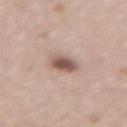Imaged during a routine full-body skin examination; the lesion was not biopsied and no histopathology is available.
Cropped from a whole-body photographic skin survey; the tile spans about 15 mm.
Approximately 3 mm at its widest.
From the back.
A female patient approximately 75 years of age.
Automated tile analysis of the lesion measured an average lesion color of about L≈54 a*≈17 b*≈24 (CIELAB), about 14 CIELAB-L* units darker than the surrounding skin, and a normalized border contrast of about 9.5. And it measured internal color variation of about 4 on a 0–10 scale and radial color variation of about 1.